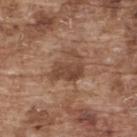biopsy status: total-body-photography surveillance lesion; no biopsy | automated lesion analysis: an area of roughly 9.5 mm², a shape eccentricity near 0.65, and a shape-asymmetry score of about 0.35 (0 = symmetric); a lesion color around L≈45 a*≈19 b*≈28 in CIELAB and roughly 10 lightness units darker than nearby skin; an automated nevus-likeness rating near 0 out of 100 and a lesion-detection confidence of about 100/100 | subject: male, roughly 75 years of age | diameter: about 4.5 mm | location: the upper back | image: total-body-photography crop, ~15 mm field of view | illumination: white-light illumination.